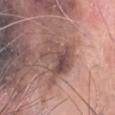Impression:
The lesion was photographed on a routine skin check and not biopsied; there is no pathology result.
Clinical summary:
About 5 mm across. From the head or neck. A male subject, about 80 years old. A region of skin cropped from a whole-body photographic capture, roughly 15 mm wide. Automated tile analysis of the lesion measured an area of roughly 13 mm² and an eccentricity of roughly 0.65. The tile uses white-light illumination.